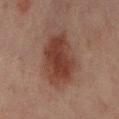Impression:
The lesion was tiled from a total-body skin photograph and was not biopsied.
Acquisition and patient details:
A 15 mm close-up extracted from a 3D total-body photography capture. About 6 mm across. The tile uses cross-polarized illumination. The patient is a female approximately 55 years of age. Automated image analysis of the tile measured a lesion area of about 22 mm² and a symmetry-axis asymmetry near 0.2. And it measured an average lesion color of about L≈34 a*≈20 b*≈23 (CIELAB) and about 10 CIELAB-L* units darker than the surrounding skin. Located on the left lower leg.